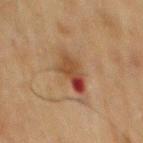{
  "biopsy_status": "not biopsied; imaged during a skin examination",
  "lesion_size": {
    "long_diameter_mm_approx": 5.0
  },
  "site": "mid back",
  "image": {
    "source": "total-body photography crop",
    "field_of_view_mm": 15
  },
  "patient": {
    "sex": "male",
    "age_approx": 70
  }
}